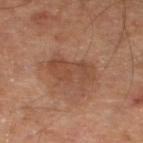illumination: cross-polarized illumination | patient: male, roughly 55 years of age | site: the left lower leg | imaging modality: 15 mm crop, total-body photography | automated lesion analysis: a mean CIELAB color near L≈43 a*≈20 b*≈28, roughly 7 lightness units darker than nearby skin, and a lesion-to-skin contrast of about 6 (normalized; higher = more distinct); a nevus-likeness score of about 0/100 | diameter: ≈5.5 mm.